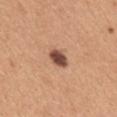Notes:
* notes — no biopsy performed (imaged during a skin exam)
* patient — female, in their 30s
* image source — total-body-photography crop, ~15 mm field of view
* anatomic site — the back